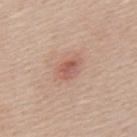The lesion was tiled from a total-body skin photograph and was not biopsied. A male patient, roughly 45 years of age. Cropped from a total-body skin-imaging series; the visible field is about 15 mm. Automated tile analysis of the lesion measured a border-irregularity index near 2.5/10, a within-lesion color-variation index near 4.5/10, and peripheral color asymmetry of about 2. And it measured a classifier nevus-likeness of about 40/100 and a lesion-detection confidence of about 100/100. The lesion is located on the mid back.